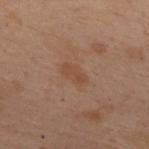Q: Is there a histopathology result?
A: total-body-photography surveillance lesion; no biopsy
Q: Lesion size?
A: ≈3 mm
Q: Who is the patient?
A: female, aged 53 to 57
Q: How was this image acquired?
A: 15 mm crop, total-body photography
Q: What is the anatomic site?
A: the upper back